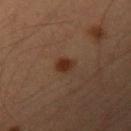<tbp_lesion>
<biopsy_status>not biopsied; imaged during a skin examination</biopsy_status>
<image>
  <source>total-body photography crop</source>
  <field_of_view_mm>15</field_of_view_mm>
</image>
<lesion_size>
  <long_diameter_mm_approx>2.5</long_diameter_mm_approx>
</lesion_size>
<site>left upper arm</site>
<patient>
  <sex>male</sex>
  <age_approx>35</age_approx>
</patient>
</tbp_lesion>